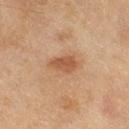Captured during whole-body skin photography for melanoma surveillance; the lesion was not biopsied.
A lesion tile, about 15 mm wide, cut from a 3D total-body photograph.
On the right thigh.
The tile uses cross-polarized illumination.
Automated image analysis of the tile measured a classifier nevus-likeness of about 75/100 and a lesion-detection confidence of about 100/100.
A female subject about 60 years old.
Longest diameter approximately 3.5 mm.